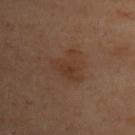Assessment: This lesion was catalogued during total-body skin photography and was not selected for biopsy. Context: This is a cross-polarized tile. The recorded lesion diameter is about 4 mm. Located on the upper back. A female patient, aged approximately 55. A 15 mm close-up tile from a total-body photography series done for melanoma screening. The lesion-visualizer software estimated a border-irregularity index near 6.5/10, a within-lesion color-variation index near 2/10, and peripheral color asymmetry of about 0.5. The software also gave a classifier nevus-likeness of about 15/100 and a detector confidence of about 100 out of 100 that the crop contains a lesion.